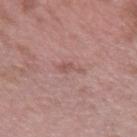The lesion was photographed on a routine skin check and not biopsied; there is no pathology result. A male subject, approximately 40 years of age. A lesion tile, about 15 mm wide, cut from a 3D total-body photograph. Longest diameter approximately 2.5 mm. From the right upper arm. An algorithmic analysis of the crop reported a lesion area of about 2.5 mm² and a symmetry-axis asymmetry near 0.45. And it measured roughly 7 lightness units darker than nearby skin and a normalized lesion–skin contrast near 5.5. The analysis additionally found a border-irregularity rating of about 5.5/10, internal color variation of about 1 on a 0–10 scale, and a peripheral color-asymmetry measure near 0.5. It also reported a classifier nevus-likeness of about 0/100.